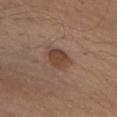Imaged during a routine full-body skin examination; the lesion was not biopsied and no histopathology is available.
The patient is a male in their mid- to late 60s.
From the chest.
A roughly 15 mm field-of-view crop from a total-body skin photograph.
Measured at roughly 3.5 mm in maximum diameter.
Automated tile analysis of the lesion measured a lesion area of about 7 mm². The analysis additionally found a mean CIELAB color near L≈43 a*≈18 b*≈28 and roughly 9 lightness units darker than nearby skin. The analysis additionally found a border-irregularity index near 1.5/10, a color-variation rating of about 2.5/10, and peripheral color asymmetry of about 1.